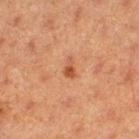Recorded during total-body skin imaging; not selected for excision or biopsy. A 15 mm close-up extracted from a 3D total-body photography capture. A female patient, approximately 40 years of age. The lesion is on the right thigh.